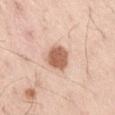The lesion was tiled from a total-body skin photograph and was not biopsied.
Automated tile analysis of the lesion measured a lesion area of about 7.5 mm², a shape eccentricity near 0.6, and two-axis asymmetry of about 0.15. The analysis additionally found a mean CIELAB color near L≈61 a*≈23 b*≈32 and a normalized lesion–skin contrast near 10.
Captured under white-light illumination.
A lesion tile, about 15 mm wide, cut from a 3D total-body photograph.
A male subject, in their mid- to late 50s.
Approximately 3.5 mm at its widest.
The lesion is on the front of the torso.